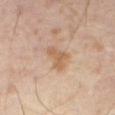The lesion was photographed on a routine skin check and not biopsied; there is no pathology result. An algorithmic analysis of the crop reported a lesion area of about 5 mm², an eccentricity of roughly 0.75, and two-axis asymmetry of about 0.5. This is a cross-polarized tile. Measured at roughly 3 mm in maximum diameter. A region of skin cropped from a whole-body photographic capture, roughly 15 mm wide. A male patient aged 63–67.